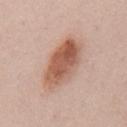{
  "biopsy_status": "not biopsied; imaged during a skin examination",
  "automated_metrics": {
    "eccentricity": 0.85,
    "border_irregularity_0_10": 2.0,
    "color_variation_0_10": 7.0,
    "peripheral_color_asymmetry": 2.5
  },
  "lighting": "white-light",
  "site": "chest",
  "image": {
    "source": "total-body photography crop",
    "field_of_view_mm": 15
  },
  "patient": {
    "sex": "male",
    "age_approx": 50
  }
}